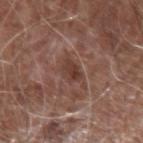Q: Was a biopsy performed?
A: total-body-photography surveillance lesion; no biopsy
Q: How large is the lesion?
A: ~2.5 mm (longest diameter)
Q: Illumination type?
A: white-light
Q: What kind of image is this?
A: 15 mm crop, total-body photography
Q: What is the anatomic site?
A: the right forearm
Q: What did automated image analysis measure?
A: an area of roughly 4 mm²; an automated nevus-likeness rating near 0 out of 100 and a detector confidence of about 100 out of 100 that the crop contains a lesion
Q: What are the patient's age and sex?
A: male, aged around 75